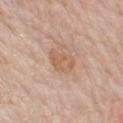The lesion was tiled from a total-body skin photograph and was not biopsied.
A male patient aged 73–77.
The lesion is located on the right upper arm.
Captured under white-light illumination.
Measured at roughly 3 mm in maximum diameter.
This image is a 15 mm lesion crop taken from a total-body photograph.